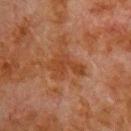<tbp_lesion>
  <biopsy_status>not biopsied; imaged during a skin examination</biopsy_status>
  <patient>
    <sex>male</sex>
    <age_approx>80</age_approx>
  </patient>
  <lesion_size>
    <long_diameter_mm_approx>4.0</long_diameter_mm_approx>
  </lesion_size>
  <lighting>cross-polarized</lighting>
  <site>front of the torso</site>
  <image>
    <source>total-body photography crop</source>
    <field_of_view_mm>15</field_of_view_mm>
  </image>
</tbp_lesion>